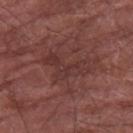Clinical impression: The lesion was tiled from a total-body skin photograph and was not biopsied. Acquisition and patient details: The lesion-visualizer software estimated a lesion color around L≈34 a*≈22 b*≈21 in CIELAB and a lesion–skin lightness drop of about 6. It also reported border irregularity of about 7 on a 0–10 scale and peripheral color asymmetry of about 1. The software also gave a nevus-likeness score of about 0/100. From the arm. A male subject aged 73 to 77. Imaged with white-light lighting. A region of skin cropped from a whole-body photographic capture, roughly 15 mm wide.